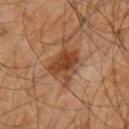This lesion was catalogued during total-body skin photography and was not selected for biopsy. Located on the left upper arm. An algorithmic analysis of the crop reported border irregularity of about 3.5 on a 0–10 scale, a color-variation rating of about 4.5/10, and radial color variation of about 1.5. A 15 mm close-up extracted from a 3D total-body photography capture. Captured under cross-polarized illumination. A male subject, aged 58–62. Measured at roughly 4 mm in maximum diameter.